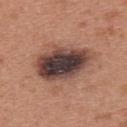Recorded during total-body skin imaging; not selected for excision or biopsy. The subject is a female approximately 40 years of age. A 15 mm close-up tile from a total-body photography series done for melanoma screening. Approximately 8 mm at its widest. This is a white-light tile. The lesion is located on the upper back.